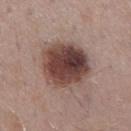Case summary:
- biopsy status — no biopsy performed (imaged during a skin exam)
- imaging modality — ~15 mm crop, total-body skin-cancer survey
- lesion diameter — ≈5.5 mm
- body site — the mid back
- tile lighting — white-light
- subject — male, in their mid-50s
- automated lesion analysis — an area of roughly 25 mm² and two-axis asymmetry of about 0.1; a lesion color around L≈43 a*≈19 b*≈21 in CIELAB, a lesion–skin lightness drop of about 16, and a normalized border contrast of about 12